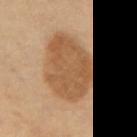Case summary:
– workup · catalogued during a skin exam; not biopsied
– location · the left thigh
– patient · female, approximately 65 years of age
– image · ~15 mm crop, total-body skin-cancer survey